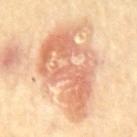notes: imaged on a skin check; not biopsied
patient: male, aged around 70
TBP lesion metrics: a mean CIELAB color near L≈69 a*≈24 b*≈35 and a normalized border contrast of about 7.5; border irregularity of about 6 on a 0–10 scale and radial color variation of about 2; a nevus-likeness score of about 20/100 and a lesion-detection confidence of about 100/100
anatomic site: the mid back
lesion size: about 9.5 mm
image: total-body-photography crop, ~15 mm field of view